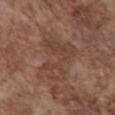Imaged during a routine full-body skin examination; the lesion was not biopsied and no histopathology is available.